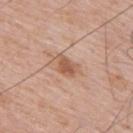<tbp_lesion>
  <biopsy_status>not biopsied; imaged during a skin examination</biopsy_status>
  <patient>
    <sex>male</sex>
    <age_approx>60</age_approx>
  </patient>
  <site>mid back</site>
  <image>
    <source>total-body photography crop</source>
    <field_of_view_mm>15</field_of_view_mm>
  </image>
</tbp_lesion>